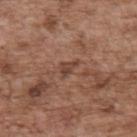Findings:
- workup — no biopsy performed (imaged during a skin exam)
- illumination — white-light illumination
- subject — male, about 70 years old
- imaging modality — total-body-photography crop, ~15 mm field of view
- size — about 2.5 mm
- location — the back